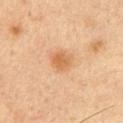biopsy status: catalogued during a skin exam; not biopsied
anatomic site: the arm
imaging modality: ~15 mm tile from a whole-body skin photo
lighting: cross-polarized
subject: male, approximately 55 years of age
diameter: ~3 mm (longest diameter)